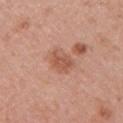The lesion was photographed on a routine skin check and not biopsied; there is no pathology result.